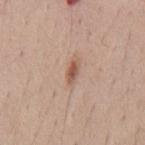Imaged during a routine full-body skin examination; the lesion was not biopsied and no histopathology is available. The lesion is located on the mid back. The subject is a male aged approximately 40. A close-up tile cropped from a whole-body skin photograph, about 15 mm across.